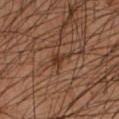Context: The lesion's longest dimension is about 3 mm. The subject is a male aged 43–47. This is a cross-polarized tile. Automated image analysis of the tile measured a lesion area of about 3.5 mm², an outline eccentricity of about 0.85 (0 = round, 1 = elongated), and two-axis asymmetry of about 0.5. And it measured a mean CIELAB color near L≈35 a*≈20 b*≈29, roughly 8 lightness units darker than nearby skin, and a lesion-to-skin contrast of about 7.5 (normalized; higher = more distinct). It also reported border irregularity of about 4.5 on a 0–10 scale and a color-variation rating of about 2/10. A 15 mm crop from a total-body photograph taken for skin-cancer surveillance. On the left forearm.